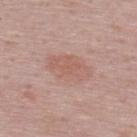Captured during whole-body skin photography for melanoma surveillance; the lesion was not biopsied. Measured at roughly 5 mm in maximum diameter. Automated tile analysis of the lesion measured a nevus-likeness score of about 0/100 and a lesion-detection confidence of about 100/100. A 15 mm close-up extracted from a 3D total-body photography capture. A male subject, about 50 years old. The lesion is located on the upper back. The tile uses white-light illumination.